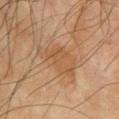  biopsy_status: not biopsied; imaged during a skin examination
  lesion_size:
    long_diameter_mm_approx: 5.5
  image:
    source: total-body photography crop
    field_of_view_mm: 15
  patient:
    sex: male
    age_approx: 75
  site: left upper arm